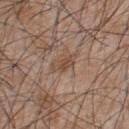workup=imaged on a skin check; not biopsied
image=~15 mm tile from a whole-body skin photo
lesion diameter=~3 mm (longest diameter)
lighting=white-light
patient=male, aged 43 to 47
site=the upper back